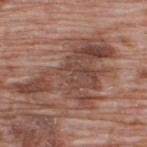follow-up: no biopsy performed (imaged during a skin exam) | automated lesion analysis: a lesion area of about 36 mm², a shape eccentricity near 0.85, and a shape-asymmetry score of about 0.6 (0 = symmetric); internal color variation of about 5.5 on a 0–10 scale | subject: male, approximately 70 years of age | image: ~15 mm crop, total-body skin-cancer survey | anatomic site: the upper back | lighting: white-light.